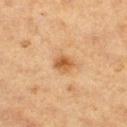Part of a total-body skin-imaging series; this lesion was reviewed on a skin check and was not flagged for biopsy.
A roughly 15 mm field-of-view crop from a total-body skin photograph.
From the right thigh.
A female patient aged around 40.
Automated tile analysis of the lesion measured a nevus-likeness score of about 85/100 and a detector confidence of about 100 out of 100 that the crop contains a lesion.
The lesion's longest dimension is about 2.5 mm.